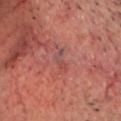Acquisition and patient details: A 15 mm crop from a total-body photograph taken for skin-cancer surveillance. Automated tile analysis of the lesion measured a mean CIELAB color near L≈47 a*≈25 b*≈24, roughly 5 lightness units darker than nearby skin, and a lesion-to-skin contrast of about 4.5 (normalized; higher = more distinct). It also reported a classifier nevus-likeness of about 0/100 and a lesion-detection confidence of about 95/100. Captured under cross-polarized illumination. The recorded lesion diameter is about 3 mm. Located on the head or neck. The subject is a male about 70 years old.